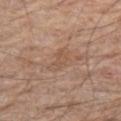Imaged during a routine full-body skin examination; the lesion was not biopsied and no histopathology is available.
A male subject aged 63–67.
The lesion is located on the leg.
A lesion tile, about 15 mm wide, cut from a 3D total-body photograph.